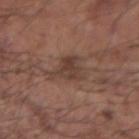Case summary:
• follow-up: total-body-photography surveillance lesion; no biopsy
• subject: male, aged 53 to 57
• body site: the left forearm
• image source: ~15 mm tile from a whole-body skin photo
• illumination: white-light